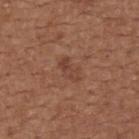Image and clinical context:
This is a white-light tile. An algorithmic analysis of the crop reported a mean CIELAB color near L≈43 a*≈22 b*≈28 and a normalized border contrast of about 5.5. The subject is a female aged around 65. From the back. A 15 mm close-up tile from a total-body photography series done for melanoma screening. About 3.5 mm across.